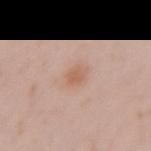Q: Was this lesion biopsied?
A: no biopsy performed (imaged during a skin exam)
Q: Where on the body is the lesion?
A: the left forearm
Q: What are the patient's age and sex?
A: female, aged around 20
Q: How was this image acquired?
A: total-body-photography crop, ~15 mm field of view